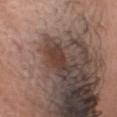Part of a total-body skin-imaging series; this lesion was reviewed on a skin check and was not flagged for biopsy. The lesion's longest dimension is about 3 mm. From the head or neck. A 15 mm close-up extracted from a 3D total-body photography capture. Imaged with white-light lighting. The subject is a male approximately 35 years of age.